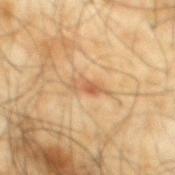| field | value |
|---|---|
| follow-up | imaged on a skin check; not biopsied |
| tile lighting | cross-polarized |
| automated metrics | an average lesion color of about L≈58 a*≈20 b*≈37 (CIELAB), a lesion–skin lightness drop of about 9, and a lesion-to-skin contrast of about 6.5 (normalized; higher = more distinct); a border-irregularity rating of about 4.5/10, internal color variation of about 4 on a 0–10 scale, and peripheral color asymmetry of about 1 |
| imaging modality | 15 mm crop, total-body photography |
| lesion size | ~4 mm (longest diameter) |
| patient | male, in their mid-60s |
| body site | the mid back |